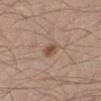This lesion was catalogued during total-body skin photography and was not selected for biopsy.
Cropped from a total-body skin-imaging series; the visible field is about 15 mm.
The lesion-visualizer software estimated an average lesion color of about L≈50 a*≈18 b*≈28 (CIELAB) and a normalized lesion–skin contrast near 8.
On the left lower leg.
The patient is a male aged approximately 35.
The lesion's longest dimension is about 2.5 mm.